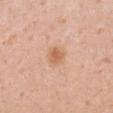Captured during whole-body skin photography for melanoma surveillance; the lesion was not biopsied.
Approximately 2.5 mm at its widest.
A roughly 15 mm field-of-view crop from a total-body skin photograph.
Captured under white-light illumination.
The subject is a male about 35 years old.
The lesion is on the right upper arm.
An algorithmic analysis of the crop reported an area of roughly 4.5 mm², an outline eccentricity of about 0.6 (0 = round, 1 = elongated), and a shape-asymmetry score of about 0.25 (0 = symmetric). It also reported a lesion color around L≈63 a*≈23 b*≈35 in CIELAB and about 9 CIELAB-L* units darker than the surrounding skin.